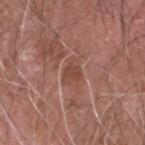Q: Was a biopsy performed?
A: no biopsy performed (imaged during a skin exam)
Q: What did automated image analysis measure?
A: an area of roughly 4 mm², an outline eccentricity of about 0.6 (0 = round, 1 = elongated), and two-axis asymmetry of about 0.35; border irregularity of about 3 on a 0–10 scale, a color-variation rating of about 2/10, and a peripheral color-asymmetry measure near 1
Q: Where on the body is the lesion?
A: the head or neck
Q: How large is the lesion?
A: ~2.5 mm (longest diameter)
Q: How was this image acquired?
A: total-body-photography crop, ~15 mm field of view
Q: What lighting was used for the tile?
A: white-light
Q: What are the patient's age and sex?
A: male, aged 73 to 77